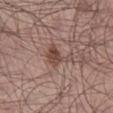patient = male, roughly 55 years of age
image = ~15 mm tile from a whole-body skin photo
illumination = white-light illumination
location = the right lower leg
diameter = ~4 mm (longest diameter)